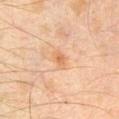follow-up = total-body-photography surveillance lesion; no biopsy
subject = male, in their 30s
image-analysis metrics = roughly 8 lightness units darker than nearby skin and a normalized border contrast of about 6
image source = 15 mm crop, total-body photography
body site = the left upper arm